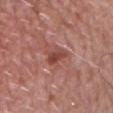Automated tile analysis of the lesion measured a shape eccentricity near 0.75 and two-axis asymmetry of about 0.3. The analysis additionally found border irregularity of about 2.5 on a 0–10 scale, internal color variation of about 3.5 on a 0–10 scale, and radial color variation of about 1.5. A 15 mm close-up tile from a total-body photography series done for melanoma screening. Captured under white-light illumination. From the upper back. The recorded lesion diameter is about 2.5 mm. The subject is a male approximately 60 years of age.